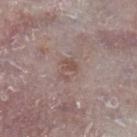Imaged during a routine full-body skin examination; the lesion was not biopsied and no histopathology is available. The lesion-visualizer software estimated an area of roughly 3.5 mm², a shape eccentricity near 0.7, and two-axis asymmetry of about 0.55. And it measured roughly 7 lightness units darker than nearby skin and a normalized border contrast of about 6. The analysis additionally found internal color variation of about 0.5 on a 0–10 scale and a peripheral color-asymmetry measure near 0. And it measured a classifier nevus-likeness of about 0/100 and lesion-presence confidence of about 100/100. The lesion's longest dimension is about 2.5 mm. A male patient, in their mid- to late 70s. A region of skin cropped from a whole-body photographic capture, roughly 15 mm wide. Located on the right lower leg. Captured under white-light illumination.